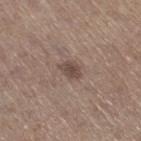Q: Was this lesion biopsied?
A: imaged on a skin check; not biopsied
Q: Who is the patient?
A: female, aged 63–67
Q: What kind of image is this?
A: 15 mm crop, total-body photography
Q: Where on the body is the lesion?
A: the right thigh
Q: Illumination type?
A: white-light illumination
Q: Lesion size?
A: ≈2.5 mm